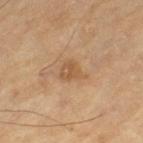Q: Was a biopsy performed?
A: catalogued during a skin exam; not biopsied
Q: Patient demographics?
A: male, aged 68 to 72
Q: What lighting was used for the tile?
A: cross-polarized
Q: What did automated image analysis measure?
A: an outline eccentricity of about 0.55 (0 = round, 1 = elongated) and two-axis asymmetry of about 0.4; a border-irregularity rating of about 4/10 and a color-variation rating of about 2.5/10; a detector confidence of about 100 out of 100 that the crop contains a lesion
Q: What is the anatomic site?
A: the left thigh
Q: What is the imaging modality?
A: total-body-photography crop, ~15 mm field of view